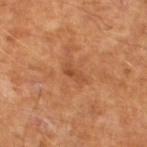Clinical impression:
Part of a total-body skin-imaging series; this lesion was reviewed on a skin check and was not flagged for biopsy.
Background:
Cropped from a total-body skin-imaging series; the visible field is about 15 mm. A male subject, about 65 years old. Automated tile analysis of the lesion measured an average lesion color of about L≈48 a*≈25 b*≈37 (CIELAB), roughly 7 lightness units darker than nearby skin, and a normalized border contrast of about 5.5. And it measured a classifier nevus-likeness of about 0/100 and lesion-presence confidence of about 100/100.